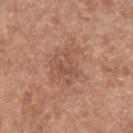Notes:
- notes · no biopsy performed (imaged during a skin exam)
- imaging modality · 15 mm crop, total-body photography
- TBP lesion metrics · a border-irregularity index near 5/10, a within-lesion color-variation index near 3.5/10, and radial color variation of about 1
- anatomic site · the left upper arm
- illumination · white-light
- subject · male, in their 60s